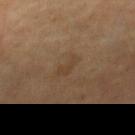biopsy status: no biopsy performed (imaged during a skin exam) | acquisition: total-body-photography crop, ~15 mm field of view | subject: female, approximately 70 years of age | body site: the right lower leg.